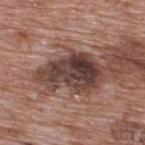biopsy status: catalogued during a skin exam; not biopsied
imaging modality: ~15 mm crop, total-body skin-cancer survey
diameter: ~6.5 mm (longest diameter)
anatomic site: the upper back
illumination: white-light illumination
patient: male, aged approximately 70